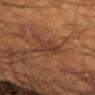Clinical impression: The lesion was photographed on a routine skin check and not biopsied; there is no pathology result. Clinical summary: A male patient approximately 65 years of age. The lesion's longest dimension is about 4.5 mm. The lesion is located on the arm. Cropped from a total-body skin-imaging series; the visible field is about 15 mm. This is a cross-polarized tile.